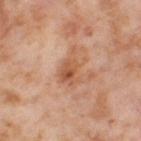Clinical impression: Part of a total-body skin-imaging series; this lesion was reviewed on a skin check and was not flagged for biopsy. Context: A female subject approximately 55 years of age. A lesion tile, about 15 mm wide, cut from a 3D total-body photograph. Located on the right thigh.